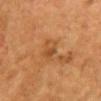Q: Patient demographics?
A: female, roughly 50 years of age
Q: What is the anatomic site?
A: the front of the torso
Q: What is the imaging modality?
A: ~15 mm crop, total-body skin-cancer survey